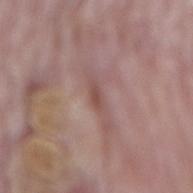No biopsy was performed on this lesion — it was imaged during a full skin examination and was not determined to be concerning.
Cropped from a total-body skin-imaging series; the visible field is about 15 mm.
The lesion is on the mid back.
A male patient aged 83 or older.
The total-body-photography lesion software estimated a border-irregularity rating of about 2.5/10 and internal color variation of about 0 on a 0–10 scale.
The tile uses white-light illumination.